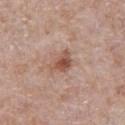Q: Was this lesion biopsied?
A: no biopsy performed (imaged during a skin exam)
Q: Who is the patient?
A: male, roughly 60 years of age
Q: What lighting was used for the tile?
A: white-light illumination
Q: Lesion size?
A: ≈3 mm
Q: What kind of image is this?
A: ~15 mm crop, total-body skin-cancer survey
Q: Automated lesion metrics?
A: an area of roughly 5.5 mm²; border irregularity of about 3.5 on a 0–10 scale, a color-variation rating of about 4/10, and a peripheral color-asymmetry measure near 1; a detector confidence of about 100 out of 100 that the crop contains a lesion
Q: Lesion location?
A: the chest